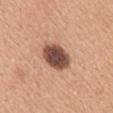biopsy status: catalogued during a skin exam; not biopsied